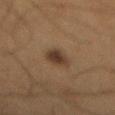lesion_size:
  long_diameter_mm_approx: 3.5
patient:
  sex: male
  age_approx: 65
lighting: cross-polarized
automated_metrics:
  area_mm2_approx: 5.5
  eccentricity: 0.85
  shape_asymmetry: 0.3
  cielab_L: 30
  cielab_a: 13
  cielab_b: 23
  vs_skin_darker_L: 9.0
  lesion_detection_confidence_0_100: 100
site: mid back
image:
  source: total-body photography crop
  field_of_view_mm: 15